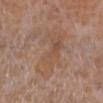No biopsy was performed on this lesion — it was imaged during a full skin examination and was not determined to be concerning. Cropped from a whole-body photographic skin survey; the tile spans about 15 mm. The subject is a female in their mid-50s. From the leg. The total-body-photography lesion software estimated a footprint of about 16 mm², an eccentricity of roughly 0.85, and a symmetry-axis asymmetry near 0.45. The analysis additionally found border irregularity of about 7.5 on a 0–10 scale, a within-lesion color-variation index near 5/10, and peripheral color asymmetry of about 1.5. The analysis additionally found an automated nevus-likeness rating near 0 out of 100 and lesion-presence confidence of about 100/100. Measured at roughly 6.5 mm in maximum diameter. This is a white-light tile.